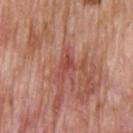Case summary:
- notes · imaged on a skin check; not biopsied
- size · about 3 mm
- lighting · white-light illumination
- subject · male, aged approximately 60
- location · the upper back
- imaging modality · ~15 mm tile from a whole-body skin photo